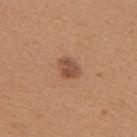Part of a total-body skin-imaging series; this lesion was reviewed on a skin check and was not flagged for biopsy.
A female subject, aged 38–42.
The total-body-photography lesion software estimated a mean CIELAB color near L≈50 a*≈22 b*≈31, roughly 10 lightness units darker than nearby skin, and a lesion-to-skin contrast of about 7.5 (normalized; higher = more distinct).
Imaged with white-light lighting.
Approximately 3 mm at its widest.
On the upper back.
A 15 mm close-up tile from a total-body photography series done for melanoma screening.